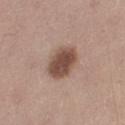{"biopsy_status": "not biopsied; imaged during a skin examination", "patient": {"sex": "female", "age_approx": 45}, "automated_metrics": {"area_mm2_approx": 11.0, "shape_asymmetry": 0.15, "border_irregularity_0_10": 1.5, "color_variation_0_10": 3.0, "peripheral_color_asymmetry": 1.0, "nevus_likeness_0_100": 75, "lesion_detection_confidence_0_100": 100}, "site": "leg", "image": {"source": "total-body photography crop", "field_of_view_mm": 15}, "lighting": "white-light"}